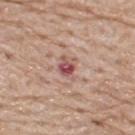| field | value |
|---|---|
| notes | total-body-photography surveillance lesion; no biopsy |
| patient | male, aged 73–77 |
| location | the upper back |
| automated metrics | a footprint of about 5 mm², an eccentricity of roughly 0.65, and a shape-asymmetry score of about 0.25 (0 = symmetric); a mean CIELAB color near L≈54 a*≈24 b*≈23 and a lesion–skin lightness drop of about 12 |
| imaging modality | ~15 mm crop, total-body skin-cancer survey |
| tile lighting | white-light illumination |
| lesion size | ~3 mm (longest diameter) |